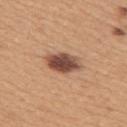{"lesion_size": {"long_diameter_mm_approx": 4.5}, "patient": {"sex": "female", "age_approx": 30}, "image": {"source": "total-body photography crop", "field_of_view_mm": 15}, "site": "right upper arm"}